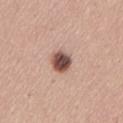notes = imaged on a skin check; not biopsied
imaging modality = total-body-photography crop, ~15 mm field of view
patient = female, aged approximately 30
anatomic site = the back
lesion size = ≈3 mm
illumination = white-light